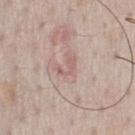Located on the chest. The patient is a male roughly 50 years of age. About 2.5 mm across. Automated tile analysis of the lesion measured a mean CIELAB color near L≈59 a*≈19 b*≈23, about 7 CIELAB-L* units darker than the surrounding skin, and a normalized lesion–skin contrast near 5. It also reported a nevus-likeness score of about 0/100 and a lesion-detection confidence of about 100/100. This image is a 15 mm lesion crop taken from a total-body photograph. This is a white-light tile.